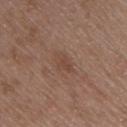Findings:
* workup · total-body-photography surveillance lesion; no biopsy
* imaging modality · total-body-photography crop, ~15 mm field of view
* subject · male, approximately 50 years of age
* illumination · white-light
* automated lesion analysis · border irregularity of about 3 on a 0–10 scale and radial color variation of about 0.5
* lesion size · ≈2.5 mm
* location · the right upper arm